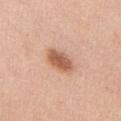Part of a total-body skin-imaging series; this lesion was reviewed on a skin check and was not flagged for biopsy.